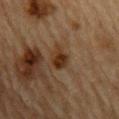Notes:
– biopsy status · total-body-photography surveillance lesion; no biopsy
– subject · male, aged 83 or over
– image source · ~15 mm tile from a whole-body skin photo
– body site · the back
– lighting · cross-polarized illumination